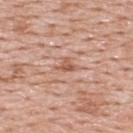This lesion was catalogued during total-body skin photography and was not selected for biopsy. The recorded lesion diameter is about 3 mm. From the upper back. This is a white-light tile. A male subject, aged around 55. Automated tile analysis of the lesion measured a lesion area of about 3 mm², an eccentricity of roughly 0.75, and two-axis asymmetry of about 0.55. The analysis additionally found an average lesion color of about L≈57 a*≈23 b*≈30 (CIELAB), roughly 9 lightness units darker than nearby skin, and a lesion-to-skin contrast of about 6.5 (normalized; higher = more distinct). And it measured a border-irregularity rating of about 5.5/10, internal color variation of about 3 on a 0–10 scale, and a peripheral color-asymmetry measure near 1. It also reported an automated nevus-likeness rating near 0 out of 100. A close-up tile cropped from a whole-body skin photograph, about 15 mm across.